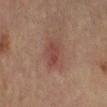{
  "biopsy_status": "not biopsied; imaged during a skin examination",
  "site": "leg",
  "image": {
    "source": "total-body photography crop",
    "field_of_view_mm": 15
  },
  "patient": {
    "sex": "female",
    "age_approx": 55
  }
}